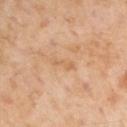Impression:
Imaged during a routine full-body skin examination; the lesion was not biopsied and no histopathology is available.
Acquisition and patient details:
This image is a 15 mm lesion crop taken from a total-body photograph. The lesion is located on the left upper arm. About 3 mm across. The total-body-photography lesion software estimated an area of roughly 3 mm², a shape eccentricity near 0.95, and a shape-asymmetry score of about 0.35 (0 = symmetric). A male patient aged 53 to 57.